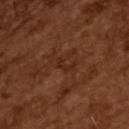Q: Was this lesion biopsied?
A: imaged on a skin check; not biopsied
Q: How was this image acquired?
A: ~15 mm tile from a whole-body skin photo
Q: Who is the patient?
A: male, in their mid- to late 60s
Q: How was the tile lit?
A: cross-polarized illumination
Q: Automated lesion metrics?
A: a lesion area of about 2 mm², a shape eccentricity near 0.9, and a shape-asymmetry score of about 0.55 (0 = symmetric); a mean CIELAB color near L≈26 a*≈23 b*≈27, a lesion–skin lightness drop of about 5, and a normalized border contrast of about 5.5; a border-irregularity index near 5.5/10, internal color variation of about 0 on a 0–10 scale, and a peripheral color-asymmetry measure near 0
Q: Lesion size?
A: about 2.5 mm